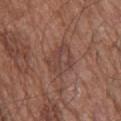| feature | finding |
|---|---|
| size | ~4 mm (longest diameter) |
| image source | total-body-photography crop, ~15 mm field of view |
| subject | male, in their mid-70s |
| location | the upper back |